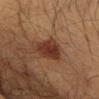follow-up: no biopsy performed (imaged during a skin exam); lesion diameter: about 4 mm; acquisition: ~15 mm crop, total-body skin-cancer survey; patient: male, aged 63 to 67; anatomic site: the chest.